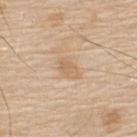Imaged during a routine full-body skin examination; the lesion was not biopsied and no histopathology is available.
A region of skin cropped from a whole-body photographic capture, roughly 15 mm wide.
About 3 mm across.
Captured under white-light illumination.
Located on the upper back.
The subject is a male aged 78 to 82.